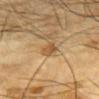Case summary:
• follow-up — no biopsy performed (imaged during a skin exam)
• anatomic site — the front of the torso
• tile lighting — cross-polarized
• acquisition — ~15 mm crop, total-body skin-cancer survey
• lesion size — about 2.5 mm
• subject — male, about 85 years old or older